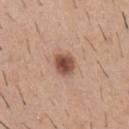notes: imaged on a skin check; not biopsied
tile lighting: white-light
imaging modality: ~15 mm tile from a whole-body skin photo
diameter: about 2.5 mm
patient: male, approximately 40 years of age
automated metrics: an area of roughly 6 mm² and a shape eccentricity near 0.45; an average lesion color of about L≈50 a*≈21 b*≈28 (CIELAB), roughly 15 lightness units darker than nearby skin, and a lesion-to-skin contrast of about 10.5 (normalized; higher = more distinct); a border-irregularity rating of about 1.5/10, internal color variation of about 4 on a 0–10 scale, and a peripheral color-asymmetry measure near 1
body site: the front of the torso